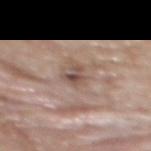Captured during whole-body skin photography for melanoma surveillance; the lesion was not biopsied.
The patient is a female in their 60s.
The total-body-photography lesion software estimated a lesion area of about 3.5 mm² and an eccentricity of roughly 0.75. And it measured an automated nevus-likeness rating near 5 out of 100 and a lesion-detection confidence of about 100/100.
This image is a 15 mm lesion crop taken from a total-body photograph.
This is a white-light tile.
The recorded lesion diameter is about 2.5 mm.
From the upper back.